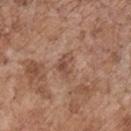A roughly 15 mm field-of-view crop from a total-body skin photograph.
A male patient roughly 70 years of age.
On the chest.
This is a white-light tile.